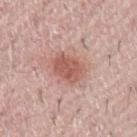The recorded lesion diameter is about 4 mm.
Automated image analysis of the tile measured an eccentricity of roughly 0.7 and a symmetry-axis asymmetry near 0.15. And it measured about 11 CIELAB-L* units darker than the surrounding skin and a normalized lesion–skin contrast near 7.5. It also reported a border-irregularity rating of about 1.5/10, a within-lesion color-variation index near 2.5/10, and peripheral color asymmetry of about 1. It also reported a nevus-likeness score of about 95/100 and lesion-presence confidence of about 100/100.
The subject is a male aged 48–52.
Located on the left upper arm.
A close-up tile cropped from a whole-body skin photograph, about 15 mm across.